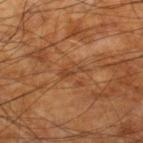biopsy status: total-body-photography surveillance lesion; no biopsy | location: the right lower leg | subject: male, in their 60s | image-analysis metrics: a lesion area of about 3 mm², a shape eccentricity near 0.9, and a symmetry-axis asymmetry near 0.35; a mean CIELAB color near L≈42 a*≈22 b*≈35 and a lesion–skin lightness drop of about 7; a classifier nevus-likeness of about 0/100 and lesion-presence confidence of about 90/100 | size: ≈3 mm | acquisition: ~15 mm tile from a whole-body skin photo | tile lighting: cross-polarized illumination.